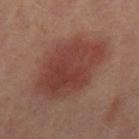Imaged during a routine full-body skin examination; the lesion was not biopsied and no histopathology is available.
Imaged with cross-polarized lighting.
Measured at roughly 9 mm in maximum diameter.
A 15 mm close-up extracted from a 3D total-body photography capture.
A female patient in their 50s.
Located on the leg.